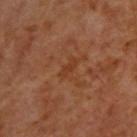The lesion was photographed on a routine skin check and not biopsied; there is no pathology result. A male subject, approximately 65 years of age. A region of skin cropped from a whole-body photographic capture, roughly 15 mm wide. Longest diameter approximately 2.5 mm. The lesion is on the upper back. Captured under cross-polarized illumination.